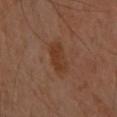The lesion was photographed on a routine skin check and not biopsied; there is no pathology result.
A 15 mm crop from a total-body photograph taken for skin-cancer surveillance.
Measured at roughly 4 mm in maximum diameter.
On the left arm.
Imaged with cross-polarized lighting.
A female subject aged 58–62.